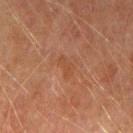The lesion was tiled from a total-body skin photograph and was not biopsied.
From the left thigh.
Longest diameter approximately 3 mm.
This is a cross-polarized tile.
A region of skin cropped from a whole-body photographic capture, roughly 15 mm wide.
The subject is a male roughly 75 years of age.
Automated tile analysis of the lesion measured an area of roughly 3.5 mm² and an eccentricity of roughly 0.85. And it measured a lesion color around L≈39 a*≈20 b*≈29 in CIELAB and roughly 4 lightness units darker than nearby skin.